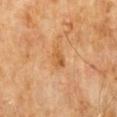  biopsy_status: not biopsied; imaged during a skin examination